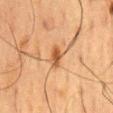Clinical impression: The lesion was tiled from a total-body skin photograph and was not biopsied. Clinical summary: Located on the mid back. A 15 mm close-up extracted from a 3D total-body photography capture. The total-body-photography lesion software estimated a lesion color around L≈44 a*≈20 b*≈33 in CIELAB, a lesion–skin lightness drop of about 10, and a normalized border contrast of about 8. A male patient aged 58 to 62.